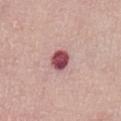notes = imaged on a skin check; not biopsied